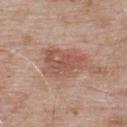Notes:
• follow-up — catalogued during a skin exam; not biopsied
• lighting — white-light
• patient — male, approximately 55 years of age
• diameter — about 5 mm
• acquisition — ~15 mm crop, total-body skin-cancer survey
• body site — the upper back
• TBP lesion metrics — an area of roughly 13 mm², an eccentricity of roughly 0.75, and two-axis asymmetry of about 0.3; a lesion color around L≈54 a*≈22 b*≈28 in CIELAB; a border-irregularity index near 3.5/10, a color-variation rating of about 3.5/10, and a peripheral color-asymmetry measure near 1.5